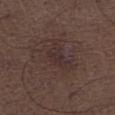follow-up = catalogued during a skin exam; not biopsied | imaging modality = ~15 mm tile from a whole-body skin photo | site = the right lower leg | diameter = ≈3 mm | subject = male, roughly 50 years of age.